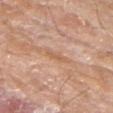Captured during whole-body skin photography for melanoma surveillance; the lesion was not biopsied. The subject is a male roughly 80 years of age. Measured at roughly 2.5 mm in maximum diameter. The lesion is on the left forearm. A 15 mm close-up extracted from a 3D total-body photography capture. The total-body-photography lesion software estimated a footprint of about 3 mm², an outline eccentricity of about 0.85 (0 = round, 1 = elongated), and a shape-asymmetry score of about 0.35 (0 = symmetric). The software also gave about 7 CIELAB-L* units darker than the surrounding skin and a lesion-to-skin contrast of about 5 (normalized; higher = more distinct). It also reported a nevus-likeness score of about 0/100.